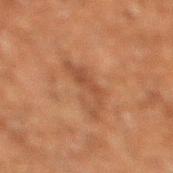Findings:
• biopsy status — imaged on a skin check; not biopsied
• site — the right lower leg
• imaging modality — 15 mm crop, total-body photography
• lesion size — about 5 mm
• tile lighting — cross-polarized
• patient — male, aged 58–62